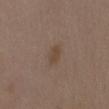<record>
  <biopsy_status>not biopsied; imaged during a skin examination</biopsy_status>
  <lesion_size>
    <long_diameter_mm_approx>2.5</long_diameter_mm_approx>
  </lesion_size>
  <site>abdomen</site>
  <image>
    <source>total-body photography crop</source>
    <field_of_view_mm>15</field_of_view_mm>
  </image>
  <patient>
    <sex>female</sex>
    <age_approx>35</age_approx>
  </patient>
</record>